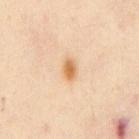Recorded during total-body skin imaging; not selected for excision or biopsy. From the chest. Longest diameter approximately 2.5 mm. This is a cross-polarized tile. A male patient, in their mid-40s. A 15 mm close-up tile from a total-body photography series done for melanoma screening.